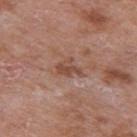Acquisition and patient details: A male subject, roughly 70 years of age. The lesion's longest dimension is about 3 mm. This is a white-light tile. Automated image analysis of the tile measured an area of roughly 4.5 mm² and a shape eccentricity near 0.7. The analysis additionally found an average lesion color of about L≈48 a*≈21 b*≈28 (CIELAB), a lesion–skin lightness drop of about 9, and a normalized lesion–skin contrast near 7. It also reported a border-irregularity rating of about 4/10, a within-lesion color-variation index near 2/10, and radial color variation of about 0.5. A 15 mm close-up tile from a total-body photography series done for melanoma screening. Located on the right upper arm.